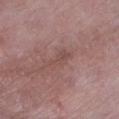| feature | finding |
|---|---|
| workup | total-body-photography surveillance lesion; no biopsy |
| automated lesion analysis | a border-irregularity index near 5.5/10 and a color-variation rating of about 1/10; a detector confidence of about 95 out of 100 that the crop contains a lesion |
| lesion diameter | about 4 mm |
| acquisition | total-body-photography crop, ~15 mm field of view |
| site | the left lower leg |
| patient | female, in their 70s |